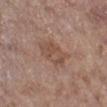biopsy_status: not biopsied; imaged during a skin examination
patient:
  sex: female
  age_approx: 85+
site: leg
image:
  source: total-body photography crop
  field_of_view_mm: 15